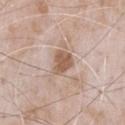– workup: total-body-photography surveillance lesion; no biopsy
– acquisition: ~15 mm tile from a whole-body skin photo
– patient: male, about 50 years old
– location: the chest